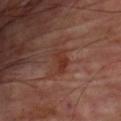biopsy status — total-body-photography surveillance lesion; no biopsy | patient — male, aged approximately 65 | anatomic site — the chest | image — ~15 mm crop, total-body skin-cancer survey.